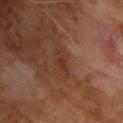Q: Lesion location?
A: the chest
Q: What is the imaging modality?
A: total-body-photography crop, ~15 mm field of view
Q: Patient demographics?
A: male, aged 58–62